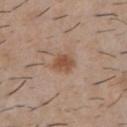Captured during whole-body skin photography for melanoma surveillance; the lesion was not biopsied. A male patient, aged 28 to 32. A 15 mm crop from a total-body photograph taken for skin-cancer surveillance. Automated image analysis of the tile measured an average lesion color of about L≈51 a*≈20 b*≈31 (CIELAB). It also reported border irregularity of about 2.5 on a 0–10 scale, a color-variation rating of about 2/10, and a peripheral color-asymmetry measure near 0.5. The lesion is located on the chest.